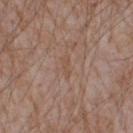The recorded lesion diameter is about 2.5 mm.
A male subject in their mid- to late 70s.
The lesion-visualizer software estimated a border-irregularity rating of about 2.5/10, a color-variation rating of about 0/10, and peripheral color asymmetry of about 0.
A lesion tile, about 15 mm wide, cut from a 3D total-body photograph.
The tile uses white-light illumination.
The lesion is located on the right upper arm.